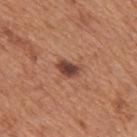Q: Is there a histopathology result?
A: catalogued during a skin exam; not biopsied
Q: Automated lesion metrics?
A: a lesion area of about 4 mm²
Q: What are the patient's age and sex?
A: male, roughly 65 years of age
Q: Where on the body is the lesion?
A: the mid back
Q: Lesion size?
A: ~3 mm (longest diameter)
Q: What kind of image is this?
A: total-body-photography crop, ~15 mm field of view
Q: How was the tile lit?
A: white-light